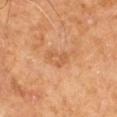Q: Was this lesion biopsied?
A: imaged on a skin check; not biopsied
Q: How large is the lesion?
A: ≈2.5 mm
Q: How was this image acquired?
A: total-body-photography crop, ~15 mm field of view
Q: What is the anatomic site?
A: the back
Q: What are the patient's age and sex?
A: male, aged approximately 60